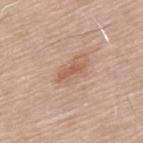Part of a total-body skin-imaging series; this lesion was reviewed on a skin check and was not flagged for biopsy. A 15 mm crop from a total-body photograph taken for skin-cancer surveillance. Located on the upper back. The patient is a male about 65 years old.